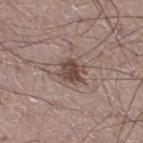Clinical impression:
The lesion was tiled from a total-body skin photograph and was not biopsied.
Clinical summary:
A roughly 15 mm field-of-view crop from a total-body skin photograph. A male subject, aged around 50. From the right thigh.